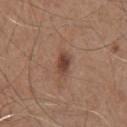biopsy_status: not biopsied; imaged during a skin examination
site: chest
patient:
  sex: male
  age_approx: 75
lesion_size:
  long_diameter_mm_approx: 3.0
image:
  source: total-body photography crop
  field_of_view_mm: 15
lighting: white-light